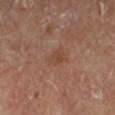• workup — no biopsy performed (imaged during a skin exam)
• anatomic site — the left lower leg
• image-analysis metrics — a within-lesion color-variation index near 2/10 and radial color variation of about 0.5; an automated nevus-likeness rating near 5 out of 100 and a lesion-detection confidence of about 100/100
• lighting — cross-polarized illumination
• image source — ~15 mm tile from a whole-body skin photo
• size — ≈2.5 mm
• patient — male, roughly 65 years of age